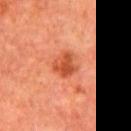Impression: Recorded during total-body skin imaging; not selected for excision or biopsy. Context: The total-body-photography lesion software estimated a footprint of about 6 mm², a shape eccentricity near 0.4, and a symmetry-axis asymmetry near 0.35. The software also gave a classifier nevus-likeness of about 80/100 and a lesion-detection confidence of about 100/100. A region of skin cropped from a whole-body photographic capture, roughly 15 mm wide. A male subject roughly 65 years of age. About 3 mm across. On the chest.